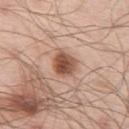Recorded during total-body skin imaging; not selected for excision or biopsy. Approximately 3.5 mm at its widest. A 15 mm close-up extracted from a 3D total-body photography capture. From the left thigh. Captured under white-light illumination. A male patient approximately 55 years of age.